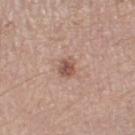Impression: No biopsy was performed on this lesion — it was imaged during a full skin examination and was not determined to be concerning. Acquisition and patient details: This is a white-light tile. Located on the right thigh. A region of skin cropped from a whole-body photographic capture, roughly 15 mm wide. The subject is a male approximately 75 years of age. Longest diameter approximately 2.5 mm. An algorithmic analysis of the crop reported an area of roughly 4 mm², an outline eccentricity of about 0.65 (0 = round, 1 = elongated), and a symmetry-axis asymmetry near 0.2. And it measured a lesion color around L≈53 a*≈20 b*≈26 in CIELAB and a lesion-to-skin contrast of about 8 (normalized; higher = more distinct). And it measured a nevus-likeness score of about 70/100.